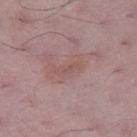{
  "biopsy_status": "not biopsied; imaged during a skin examination",
  "automated_metrics": {
    "cielab_L": 52,
    "cielab_a": 20,
    "cielab_b": 22,
    "vs_skin_darker_L": 5.0,
    "vs_skin_contrast_norm": 5.0,
    "lesion_detection_confidence_0_100": 95
  },
  "image": {
    "source": "total-body photography crop",
    "field_of_view_mm": 15
  },
  "patient": {
    "sex": "male",
    "age_approx": 50
  },
  "site": "right thigh",
  "lesion_size": {
    "long_diameter_mm_approx": 3.0
  }
}